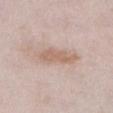Notes:
• notes: catalogued during a skin exam; not biopsied
• image source: ~15 mm tile from a whole-body skin photo
• subject: female, aged 63 to 67
• body site: the chest
• lesion size: ~5 mm (longest diameter)
• lighting: white-light